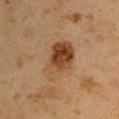site — the right upper arm
subject — male, aged around 45
image source — 15 mm crop, total-body photography
lighting — cross-polarized illumination